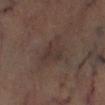The tile uses cross-polarized illumination.
Approximately 4 mm at its widest.
A male patient in their 70s.
An algorithmic analysis of the crop reported a color-variation rating of about 2.5/10 and radial color variation of about 1. It also reported a classifier nevus-likeness of about 0/100.
Cropped from a total-body skin-imaging series; the visible field is about 15 mm.
From the left lower leg.